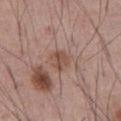biopsy_status: not biopsied; imaged during a skin examination